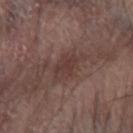Background: A male subject, roughly 75 years of age. Captured under white-light illumination. On the arm. An algorithmic analysis of the crop reported border irregularity of about 4.5 on a 0–10 scale. A 15 mm crop from a total-body photograph taken for skin-cancer surveillance. The lesion's longest dimension is about 5 mm.